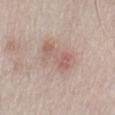workup = no biopsy performed (imaged during a skin exam); lighting = white-light; diameter = ≈5.5 mm; site = the left lower leg; patient = female, aged 58–62; image source = ~15 mm tile from a whole-body skin photo.